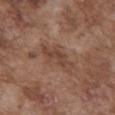Acquisition and patient details: Imaged with white-light lighting. The lesion's longest dimension is about 5 mm. A 15 mm close-up extracted from a 3D total-body photography capture. The lesion is on the abdomen. An algorithmic analysis of the crop reported a lesion color around L≈43 a*≈19 b*≈27 in CIELAB, roughly 8 lightness units darker than nearby skin, and a normalized lesion–skin contrast near 6. It also reported a color-variation rating of about 2/10 and a peripheral color-asymmetry measure near 0.5. The subject is a male approximately 75 years of age.